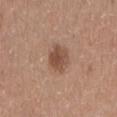Recorded during total-body skin imaging; not selected for excision or biopsy. Imaged with white-light lighting. A 15 mm close-up extracted from a 3D total-body photography capture. A male patient in their 20s. About 3.5 mm across. The lesion is on the mid back.